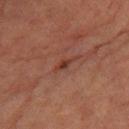{
  "biopsy_status": "not biopsied; imaged during a skin examination",
  "lesion_size": {
    "long_diameter_mm_approx": 2.5
  },
  "automated_metrics": {
    "border_irregularity_0_10": 3.5,
    "color_variation_0_10": 2.0,
    "peripheral_color_asymmetry": 0.5
  },
  "image": {
    "source": "total-body photography crop",
    "field_of_view_mm": 15
  },
  "lighting": "cross-polarized",
  "site": "right thigh",
  "patient": {
    "sex": "male",
    "age_approx": "85+"
  }
}